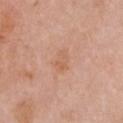The lesion was tiled from a total-body skin photograph and was not biopsied.
The lesion is located on the chest.
About 3 mm across.
This image is a 15 mm lesion crop taken from a total-body photograph.
Captured under white-light illumination.
The subject is a female roughly 65 years of age.